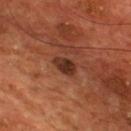No biopsy was performed on this lesion — it was imaged during a full skin examination and was not determined to be concerning.
A male patient aged 53–57.
The recorded lesion diameter is about 3 mm.
A region of skin cropped from a whole-body photographic capture, roughly 15 mm wide.
The lesion is on the chest.
The lesion-visualizer software estimated a border-irregularity rating of about 2.5/10 and a color-variation rating of about 3/10. It also reported a nevus-likeness score of about 25/100 and a detector confidence of about 100 out of 100 that the crop contains a lesion.